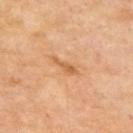This lesion was catalogued during total-body skin photography and was not selected for biopsy. Approximately 3.5 mm at its widest. This is a cross-polarized tile. The lesion is on the upper back. A male patient aged 68 to 72. A lesion tile, about 15 mm wide, cut from a 3D total-body photograph.